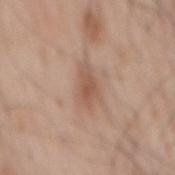{
  "biopsy_status": "not biopsied; imaged during a skin examination",
  "automated_metrics": {
    "area_mm2_approx": 7.0,
    "eccentricity": 0.85,
    "shape_asymmetry": 0.3,
    "nevus_likeness_0_100": 0
  },
  "site": "mid back",
  "image": {
    "source": "total-body photography crop",
    "field_of_view_mm": 15
  },
  "patient": {
    "sex": "male",
    "age_approx": 70
  }
}